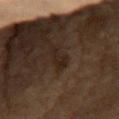biopsy_status: not biopsied; imaged during a skin examination
image:
  source: total-body photography crop
  field_of_view_mm: 15
patient:
  sex: male
  age_approx: 85+
site: front of the torso